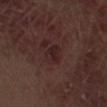No biopsy was performed on this lesion — it was imaged during a full skin examination and was not determined to be concerning.
The tile uses white-light illumination.
The recorded lesion diameter is about 3 mm.
A male subject, aged around 70.
Automated tile analysis of the lesion measured a mean CIELAB color near L≈22 a*≈18 b*≈15, roughly 6 lightness units darker than nearby skin, and a normalized lesion–skin contrast near 7.5. It also reported a lesion-detection confidence of about 100/100.
The lesion is on the right thigh.
Cropped from a total-body skin-imaging series; the visible field is about 15 mm.